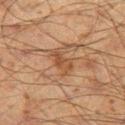<lesion>
  <biopsy_status>not biopsied; imaged during a skin examination</biopsy_status>
  <image>
    <source>total-body photography crop</source>
    <field_of_view_mm>15</field_of_view_mm>
  </image>
  <lesion_size>
    <long_diameter_mm_approx>3.5</long_diameter_mm_approx>
  </lesion_size>
  <site>left thigh</site>
  <patient>
    <sex>male</sex>
    <age_approx>60</age_approx>
  </patient>
</lesion>